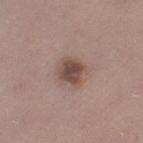  biopsy_status: not biopsied; imaged during a skin examination
  image:
    source: total-body photography crop
    field_of_view_mm: 15
  patient:
    sex: female
    age_approx: 35
  lesion_size:
    long_diameter_mm_approx: 3.0
  site: left thigh
  automated_metrics:
    color_variation_0_10: 3.5
    nevus_likeness_0_100: 55
    lesion_detection_confidence_0_100: 100